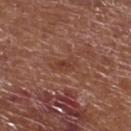follow-up = total-body-photography surveillance lesion; no biopsy
size = about 3 mm
imaging modality = 15 mm crop, total-body photography
subject = male, roughly 75 years of age
automated lesion analysis = an area of roughly 4 mm², an eccentricity of roughly 0.85, and a symmetry-axis asymmetry near 0.3; a nevus-likeness score of about 0/100 and a detector confidence of about 100 out of 100 that the crop contains a lesion
illumination = white-light illumination
site = the left lower leg